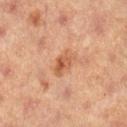workup=imaged on a skin check; not biopsied
subject=female, in their 40s
image=total-body-photography crop, ~15 mm field of view
size=≈3 mm
site=the right lower leg